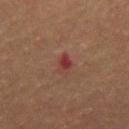  biopsy_status: not biopsied; imaged during a skin examination
  image:
    source: total-body photography crop
    field_of_view_mm: 15
  site: mid back
  patient:
    sex: male
    age_approx: 55